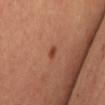Imaged during a routine full-body skin examination; the lesion was not biopsied and no histopathology is available. Cropped from a total-body skin-imaging series; the visible field is about 15 mm. A female patient, about 40 years old. From the chest.